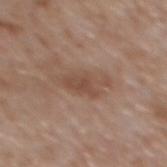Q: Was this lesion biopsied?
A: catalogued during a skin exam; not biopsied
Q: What is the imaging modality?
A: total-body-photography crop, ~15 mm field of view
Q: Lesion location?
A: the back
Q: What lighting was used for the tile?
A: white-light illumination
Q: What did automated image analysis measure?
A: a footprint of about 9 mm² and a symmetry-axis asymmetry near 0.3; a mean CIELAB color near L≈49 a*≈18 b*≈27, roughly 8 lightness units darker than nearby skin, and a normalized lesion–skin contrast near 6; a classifier nevus-likeness of about 0/100 and lesion-presence confidence of about 100/100
Q: Patient demographics?
A: male, roughly 65 years of age
Q: What is the lesion's diameter?
A: ~5 mm (longest diameter)